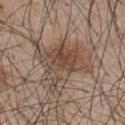{"biopsy_status": "not biopsied; imaged during a skin examination", "lighting": "white-light", "image": {"source": "total-body photography crop", "field_of_view_mm": 15}, "patient": {"sex": "male", "age_approx": 45}, "lesion_size": {"long_diameter_mm_approx": 8.5}, "site": "upper back"}